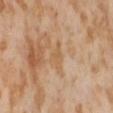notes = imaged on a skin check; not biopsied | subject = female, about 55 years old | location = the abdomen | image = ~15 mm crop, total-body skin-cancer survey.